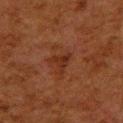notes: no biopsy performed (imaged during a skin exam)
image source: ~15 mm tile from a whole-body skin photo
tile lighting: cross-polarized
patient: male, aged approximately 80
lesion size: about 2.5 mm
anatomic site: the right lower leg